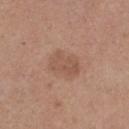| field | value |
|---|---|
| follow-up | total-body-photography surveillance lesion; no biopsy |
| anatomic site | the left thigh |
| image source | ~15 mm crop, total-body skin-cancer survey |
| patient | female, in their mid-40s |